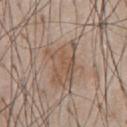Part of a total-body skin-imaging series; this lesion was reviewed on a skin check and was not flagged for biopsy. From the abdomen. A male subject about 60 years old. A 15 mm crop from a total-body photograph taken for skin-cancer surveillance.